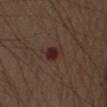Impression: Imaged during a routine full-body skin examination; the lesion was not biopsied and no histopathology is available.